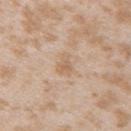This image is a 15 mm lesion crop taken from a total-body photograph. The tile uses white-light illumination. The lesion is located on the arm. The subject is a female aged 23 to 27. Automated tile analysis of the lesion measured an area of roughly 4 mm², a shape eccentricity near 0.7, and a symmetry-axis asymmetry near 0.4. The analysis additionally found a nevus-likeness score of about 0/100 and lesion-presence confidence of about 100/100.